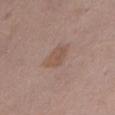workup = catalogued during a skin exam; not biopsied | patient = female, approximately 50 years of age | body site = the abdomen | image source = 15 mm crop, total-body photography.